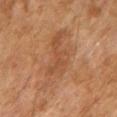Impression:
Imaged during a routine full-body skin examination; the lesion was not biopsied and no histopathology is available.
Acquisition and patient details:
The lesion's longest dimension is about 7 mm. The lesion is located on the right upper arm. Cropped from a total-body skin-imaging series; the visible field is about 15 mm. The patient is a female roughly 60 years of age. The total-body-photography lesion software estimated an area of roughly 19 mm² and a shape-asymmetry score of about 0.4 (0 = symmetric). It also reported an average lesion color of about L≈44 a*≈20 b*≈31 (CIELAB), about 6 CIELAB-L* units darker than the surrounding skin, and a normalized border contrast of about 5. And it measured a border-irregularity rating of about 6.5/10, internal color variation of about 3.5 on a 0–10 scale, and a peripheral color-asymmetry measure near 1. Captured under cross-polarized illumination.